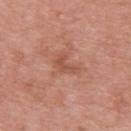Case summary:
* patient — female, aged 68 to 72
* body site — the upper back
* illumination — white-light illumination
* acquisition — total-body-photography crop, ~15 mm field of view